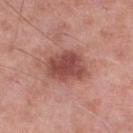Assessment:
Part of a total-body skin-imaging series; this lesion was reviewed on a skin check and was not flagged for biopsy.
Acquisition and patient details:
Located on the leg. A male subject aged 53–57. The lesion-visualizer software estimated an area of roughly 14 mm², an eccentricity of roughly 0.7, and two-axis asymmetry of about 0.2. The analysis additionally found an automated nevus-likeness rating near 50 out of 100. A roughly 15 mm field-of-view crop from a total-body skin photograph. Captured under white-light illumination. The lesion's longest dimension is about 5 mm.